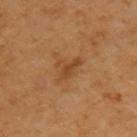| key | value |
|---|---|
| biopsy status | imaged on a skin check; not biopsied |